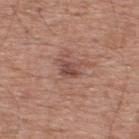Clinical impression:
Imaged during a routine full-body skin examination; the lesion was not biopsied and no histopathology is available.
Acquisition and patient details:
Automated image analysis of the tile measured a lesion area of about 3.5 mm², a shape eccentricity near 0.75, and a shape-asymmetry score of about 0.3 (0 = symmetric). The analysis additionally found a lesion color around L≈47 a*≈22 b*≈24 in CIELAB, a lesion–skin lightness drop of about 10, and a normalized border contrast of about 7.5. It also reported a border-irregularity rating of about 3/10, internal color variation of about 3 on a 0–10 scale, and peripheral color asymmetry of about 1. The recorded lesion diameter is about 2.5 mm. A male patient, in their mid-50s. A 15 mm crop from a total-body photograph taken for skin-cancer surveillance. This is a white-light tile. On the upper back.